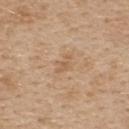Assessment:
Captured during whole-body skin photography for melanoma surveillance; the lesion was not biopsied.
Image and clinical context:
Captured under white-light illumination. From the upper back. This image is a 15 mm lesion crop taken from a total-body photograph. A female patient aged approximately 45.